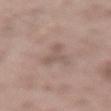The lesion was tiled from a total-body skin photograph and was not biopsied. This image is a 15 mm lesion crop taken from a total-body photograph. Imaged with white-light lighting. Automated image analysis of the tile measured a lesion area of about 4.5 mm², a shape eccentricity near 0.9, and two-axis asymmetry of about 0.7. The software also gave an average lesion color of about L≈54 a*≈16 b*≈22 (CIELAB), roughly 7 lightness units darker than nearby skin, and a lesion-to-skin contrast of about 5 (normalized; higher = more distinct). It also reported a border-irregularity rating of about 8/10 and peripheral color asymmetry of about 0.5. It also reported an automated nevus-likeness rating near 0 out of 100 and a lesion-detection confidence of about 65/100. The lesion's longest dimension is about 4 mm. A male subject, roughly 55 years of age. From the lower back.